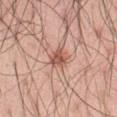Clinical summary: A male subject aged approximately 70. Cropped from a total-body skin-imaging series; the visible field is about 15 mm. From the abdomen. Imaged with white-light lighting. The lesion's longest dimension is about 3 mm. Automated image analysis of the tile measured a footprint of about 5.5 mm² and a shape-asymmetry score of about 0.2 (0 = symmetric). The analysis additionally found a nevus-likeness score of about 80/100 and a detector confidence of about 100 out of 100 that the crop contains a lesion.